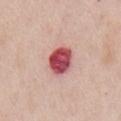Impression: Part of a total-body skin-imaging series; this lesion was reviewed on a skin check and was not flagged for biopsy. Context: The tile uses white-light illumination. From the front of the torso. Longest diameter approximately 4 mm. A 15 mm crop from a total-body photograph taken for skin-cancer surveillance. An algorithmic analysis of the crop reported about 20 CIELAB-L* units darker than the surrounding skin and a lesion-to-skin contrast of about 13 (normalized; higher = more distinct). A male patient approximately 65 years of age.